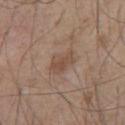Recorded during total-body skin imaging; not selected for excision or biopsy. The recorded lesion diameter is about 3.5 mm. A male patient, aged 53 to 57. Imaged with white-light lighting. This image is a 15 mm lesion crop taken from a total-body photograph. The lesion is located on the chest.